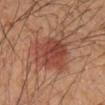Context:
Automated tile analysis of the lesion measured an outline eccentricity of about 0.8 (0 = round, 1 = elongated) and a symmetry-axis asymmetry near 0.35. It also reported an average lesion color of about L≈40 a*≈23 b*≈26 (CIELAB), about 9 CIELAB-L* units darker than the surrounding skin, and a normalized lesion–skin contrast near 7.5. The software also gave a nevus-likeness score of about 100/100 and a detector confidence of about 100 out of 100 that the crop contains a lesion. A roughly 15 mm field-of-view crop from a total-body skin photograph. The lesion is located on the left forearm. A male subject, aged 48 to 52. This is a cross-polarized tile. Approximately 8 mm at its widest.